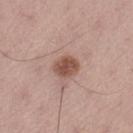| key | value |
|---|---|
| follow-up | no biopsy performed (imaged during a skin exam) |
| illumination | white-light |
| acquisition | ~15 mm crop, total-body skin-cancer survey |
| automated lesion analysis | an area of roughly 6.5 mm² and two-axis asymmetry of about 0.1; roughly 13 lightness units darker than nearby skin and a normalized border contrast of about 9; a border-irregularity rating of about 1/10, a color-variation rating of about 2.5/10, and radial color variation of about 1 |
| diameter | about 3.5 mm |
| location | the leg |
| subject | male, approximately 55 years of age |